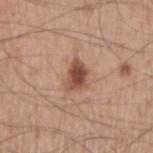follow-up: no biopsy performed (imaged during a skin exam) | image-analysis metrics: a border-irregularity rating of about 3/10, internal color variation of about 5 on a 0–10 scale, and a peripheral color-asymmetry measure near 1.5 | subject: male, approximately 55 years of age | anatomic site: the right upper arm | image: 15 mm crop, total-body photography.